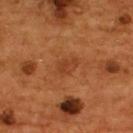workup — total-body-photography surveillance lesion; no biopsy | imaging modality — ~15 mm crop, total-body skin-cancer survey | tile lighting — cross-polarized illumination | body site — the upper back | automated lesion analysis — a border-irregularity rating of about 2.5/10 and peripheral color asymmetry of about 0.5; a nevus-likeness score of about 0/100 and a detector confidence of about 100 out of 100 that the crop contains a lesion | patient — male, in their mid- to late 50s.